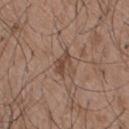Imaged during a routine full-body skin examination; the lesion was not biopsied and no histopathology is available.
A male patient aged 48–52.
On the upper back.
A 15 mm close-up extracted from a 3D total-body photography capture.
Approximately 3 mm at its widest.
This is a white-light tile.